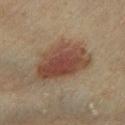Assessment:
Recorded during total-body skin imaging; not selected for excision or biopsy.
Acquisition and patient details:
Located on the left lower leg. Imaged with cross-polarized lighting. A female subject, in their mid- to late 50s. Measured at roughly 7 mm in maximum diameter. A roughly 15 mm field-of-view crop from a total-body skin photograph.